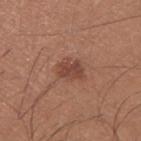Clinical impression: The lesion was tiled from a total-body skin photograph and was not biopsied. Background: Cropped from a whole-body photographic skin survey; the tile spans about 15 mm. A male subject, aged approximately 25. Located on the right upper arm. Approximately 3 mm at its widest. Captured under white-light illumination.